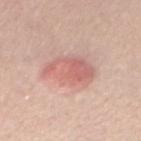| feature | finding |
|---|---|
| biopsy status | catalogued during a skin exam; not biopsied |
| tile lighting | white-light |
| patient | male, approximately 40 years of age |
| automated metrics | a color-variation rating of about 4.5/10 and radial color variation of about 1.5 |
| acquisition | total-body-photography crop, ~15 mm field of view |
| location | the arm |